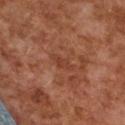No biopsy was performed on this lesion — it was imaged during a full skin examination and was not determined to be concerning.